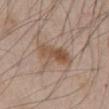• follow-up — total-body-photography surveillance lesion; no biopsy
• size — ≈4.5 mm
• location — the abdomen
• illumination — white-light illumination
• TBP lesion metrics — a lesion area of about 8 mm², a shape eccentricity near 0.9, and a shape-asymmetry score of about 0.25 (0 = symmetric); an average lesion color of about L≈51 a*≈17 b*≈29 (CIELAB) and a lesion-to-skin contrast of about 8 (normalized; higher = more distinct); a border-irregularity rating of about 3/10 and a within-lesion color-variation index near 5.5/10; an automated nevus-likeness rating near 75 out of 100 and a lesion-detection confidence of about 100/100
• image source — ~15 mm tile from a whole-body skin photo
• patient — male, roughly 45 years of age